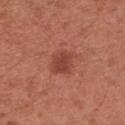On the arm.
A female subject aged 48–52.
A roughly 15 mm field-of-view crop from a total-body skin photograph.